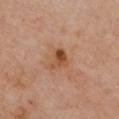Captured during whole-body skin photography for melanoma surveillance; the lesion was not biopsied.
On the chest.
Imaged with cross-polarized lighting.
The patient is a female roughly 60 years of age.
A lesion tile, about 15 mm wide, cut from a 3D total-body photograph.
The total-body-photography lesion software estimated a mean CIELAB color near L≈49 a*≈24 b*≈36, about 11 CIELAB-L* units darker than the surrounding skin, and a normalized lesion–skin contrast near 8.5. The analysis additionally found a border-irregularity index near 4.5/10.
About 3 mm across.